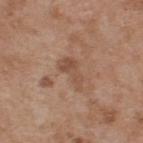Q: Is there a histopathology result?
A: catalogued during a skin exam; not biopsied
Q: How was this image acquired?
A: total-body-photography crop, ~15 mm field of view
Q: How was the tile lit?
A: white-light
Q: What are the patient's age and sex?
A: male, aged around 55
Q: What did automated image analysis measure?
A: a classifier nevus-likeness of about 0/100 and a detector confidence of about 100 out of 100 that the crop contains a lesion
Q: What is the anatomic site?
A: the back